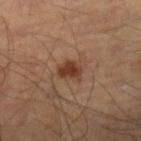Recorded during total-body skin imaging; not selected for excision or biopsy.
Automated image analysis of the tile measured a footprint of about 4.5 mm², an eccentricity of roughly 0.75, and a shape-asymmetry score of about 0.3 (0 = symmetric). The software also gave an average lesion color of about L≈38 a*≈21 b*≈29 (CIELAB), about 11 CIELAB-L* units darker than the surrounding skin, and a normalized border contrast of about 9.5. The analysis additionally found a nevus-likeness score of about 95/100 and lesion-presence confidence of about 100/100.
The lesion's longest dimension is about 3 mm.
On the left leg.
A male patient aged 48 to 52.
Cropped from a whole-body photographic skin survey; the tile spans about 15 mm.
Imaged with cross-polarized lighting.